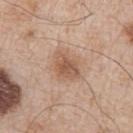| field | value |
|---|---|
| follow-up | imaged on a skin check; not biopsied |
| patient | male, about 65 years old |
| location | the arm |
| image | ~15 mm crop, total-body skin-cancer survey |